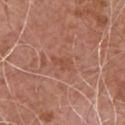* workup — total-body-photography surveillance lesion; no biopsy
* imaging modality — total-body-photography crop, ~15 mm field of view
* illumination — white-light illumination
* subject — male, aged around 55
* body site — the chest
* diameter — about 2.5 mm